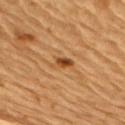notes = no biopsy performed (imaged during a skin exam); subject = male, aged 83 or over; lesion diameter = about 3.5 mm; image = total-body-photography crop, ~15 mm field of view; site = the upper back; illumination = cross-polarized.